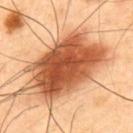Clinical impression:
Part of a total-body skin-imaging series; this lesion was reviewed on a skin check and was not flagged for biopsy.
Clinical summary:
The lesion-visualizer software estimated an area of roughly 42 mm², an outline eccentricity of about 0.75 (0 = round, 1 = elongated), and a symmetry-axis asymmetry near 0.2. It also reported a mean CIELAB color near L≈56 a*≈29 b*≈40 and a lesion–skin lightness drop of about 21. The software also gave an automated nevus-likeness rating near 95 out of 100. The tile uses cross-polarized illumination. A male subject roughly 40 years of age. Cropped from a whole-body photographic skin survey; the tile spans about 15 mm. The lesion is located on the upper back.